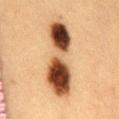Part of a total-body skin-imaging series; this lesion was reviewed on a skin check and was not flagged for biopsy. This image is a 15 mm lesion crop taken from a total-body photograph. The lesion is on the mid back. The patient is a female approximately 40 years of age. The tile uses cross-polarized illumination.